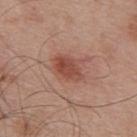Clinical impression:
The lesion was photographed on a routine skin check and not biopsied; there is no pathology result.
Image and clinical context:
From the upper back. Automated tile analysis of the lesion measured a lesion color around L≈48 a*≈25 b*≈28 in CIELAB, roughly 10 lightness units darker than nearby skin, and a normalized border contrast of about 7.5. And it measured border irregularity of about 2.5 on a 0–10 scale and radial color variation of about 1. This image is a 15 mm lesion crop taken from a total-body photograph. A male patient, approximately 55 years of age. Imaged with white-light lighting. The lesion's longest dimension is about 4 mm.